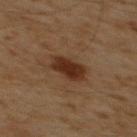follow-up = imaged on a skin check; not biopsied
image = 15 mm crop, total-body photography
subject = male, aged 58 to 62
lighting = cross-polarized illumination
lesion size = ~4 mm (longest diameter)
automated metrics = an area of roughly 8.5 mm², a shape eccentricity near 0.75, and two-axis asymmetry of about 0.2; a lesion color around L≈27 a*≈18 b*≈26 in CIELAB, roughly 10 lightness units darker than nearby skin, and a normalized border contrast of about 10.5
body site = the mid back